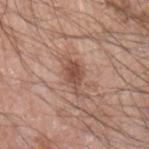Case summary:
• follow-up: catalogued during a skin exam; not biopsied
• image-analysis metrics: a footprint of about 5.5 mm², a shape eccentricity near 0.85, and a shape-asymmetry score of about 0.35 (0 = symmetric); a mean CIELAB color near L≈50 a*≈22 b*≈27 and a normalized lesion–skin contrast near 8; border irregularity of about 4.5 on a 0–10 scale, a color-variation rating of about 2/10, and a peripheral color-asymmetry measure near 0.5
• illumination: white-light illumination
• image: 15 mm crop, total-body photography
• diameter: about 4 mm
• anatomic site: the left upper arm
• patient: male, roughly 70 years of age